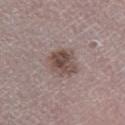{"biopsy_status": "not biopsied; imaged during a skin examination", "image": {"source": "total-body photography crop", "field_of_view_mm": 15}, "site": "leg", "patient": {"sex": "male", "age_approx": 70}, "automated_metrics": {"area_mm2_approx": 9.5, "eccentricity": 0.45, "shape_asymmetry": 0.2}, "lesion_size": {"long_diameter_mm_approx": 3.5}, "lighting": "white-light"}